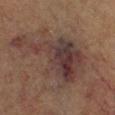Assessment: Part of a total-body skin-imaging series; this lesion was reviewed on a skin check and was not flagged for biopsy. Background: The tile uses cross-polarized illumination. On the left lower leg. The lesion's longest dimension is about 10 mm. The patient is a male aged around 85 or older. A region of skin cropped from a whole-body photographic capture, roughly 15 mm wide.